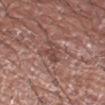Clinical summary: A male patient, aged approximately 75. The lesion is on the arm. A roughly 15 mm field-of-view crop from a total-body skin photograph. Imaged with white-light lighting. An algorithmic analysis of the crop reported a footprint of about 4.5 mm², an eccentricity of roughly 0.75, and a symmetry-axis asymmetry near 0.5. It also reported border irregularity of about 5 on a 0–10 scale, a color-variation rating of about 1.5/10, and peripheral color asymmetry of about 0.5.